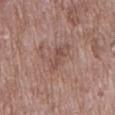Q: Was a biopsy performed?
A: total-body-photography surveillance lesion; no biopsy
Q: Lesion location?
A: the right upper arm
Q: Patient demographics?
A: female, aged 68 to 72
Q: How was this image acquired?
A: 15 mm crop, total-body photography